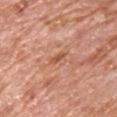| field | value |
|---|---|
| workup | catalogued during a skin exam; not biopsied |
| tile lighting | white-light illumination |
| image source | total-body-photography crop, ~15 mm field of view |
| subject | male, about 80 years old |
| site | the chest |
| diameter | about 2.5 mm |